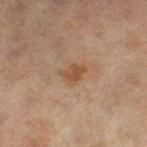Q: Is there a histopathology result?
A: total-body-photography surveillance lesion; no biopsy
Q: Where on the body is the lesion?
A: the left thigh
Q: What kind of image is this?
A: ~15 mm tile from a whole-body skin photo
Q: What are the patient's age and sex?
A: female, aged around 60
Q: Lesion size?
A: ~3 mm (longest diameter)